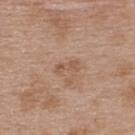Q: Is there a histopathology result?
A: no biopsy performed (imaged during a skin exam)
Q: What is the imaging modality?
A: ~15 mm crop, total-body skin-cancer survey
Q: Illumination type?
A: white-light
Q: What is the anatomic site?
A: the upper back
Q: What are the patient's age and sex?
A: female, aged 38 to 42
Q: What did automated image analysis measure?
A: a lesion area of about 3 mm² and a symmetry-axis asymmetry near 0.3; an average lesion color of about L≈54 a*≈19 b*≈31 (CIELAB) and a normalized lesion–skin contrast near 5.5; a border-irregularity rating of about 3.5/10, a within-lesion color-variation index near 0/10, and peripheral color asymmetry of about 0